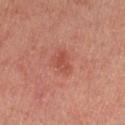The lesion was photographed on a routine skin check and not biopsied; there is no pathology result. A female subject. A 15 mm close-up extracted from a 3D total-body photography capture. Automated tile analysis of the lesion measured a shape eccentricity near 0.6 and a shape-asymmetry score of about 0.3 (0 = symmetric). It also reported a mean CIELAB color near L≈52 a*≈31 b*≈31, about 7 CIELAB-L* units darker than the surrounding skin, and a normalized border contrast of about 5.5. It also reported a border-irregularity rating of about 3/10, a within-lesion color-variation index near 3/10, and a peripheral color-asymmetry measure near 1. And it measured a classifier nevus-likeness of about 35/100 and a lesion-detection confidence of about 100/100. Imaged with cross-polarized lighting. Located on the left thigh. The recorded lesion diameter is about 2.5 mm.